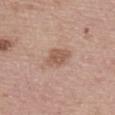biopsy status — imaged on a skin check; not biopsied | image — 15 mm crop, total-body photography | patient — female, aged around 65 | location — the back | automated metrics — an average lesion color of about L≈55 a*≈20 b*≈28 (CIELAB); a border-irregularity index near 2.5/10, a color-variation rating of about 1.5/10, and radial color variation of about 0.5 | lighting — white-light illumination | size — about 2.5 mm.